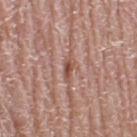Q: What are the patient's age and sex?
A: female, in their mid-60s
Q: What kind of image is this?
A: 15 mm crop, total-body photography
Q: What lighting was used for the tile?
A: white-light
Q: How large is the lesion?
A: about 3 mm
Q: What is the anatomic site?
A: the leg